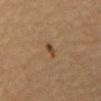Clinical impression: This lesion was catalogued during total-body skin photography and was not selected for biopsy. Background: A male subject, aged 58–62. The tile uses cross-polarized illumination. A 15 mm close-up extracted from a 3D total-body photography capture. The lesion is located on the mid back.